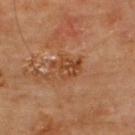<tbp_lesion>
<biopsy_status>not biopsied; imaged during a skin examination</biopsy_status>
<lighting>cross-polarized</lighting>
<automated_metrics>
  <area_mm2_approx>7.0</area_mm2_approx>
  <eccentricity>0.6</eccentricity>
  <shape_asymmetry>0.3</shape_asymmetry>
  <border_irregularity_0_10>4.0</border_irregularity_0_10>
  <color_variation_0_10>4.5</color_variation_0_10>
  <peripheral_color_asymmetry>1.5</peripheral_color_asymmetry>
  <nevus_likeness_0_100>0</nevus_likeness_0_100>
  <lesion_detection_confidence_0_100>100</lesion_detection_confidence_0_100>
</automated_metrics>
<image>
  <source>total-body photography crop</source>
  <field_of_view_mm>15</field_of_view_mm>
</image>
<site>back</site>
<patient>
  <sex>male</sex>
  <age_approx>65</age_approx>
</patient>
</tbp_lesion>